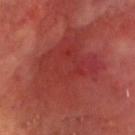workup: total-body-photography surveillance lesion; no biopsy
anatomic site: the head or neck
subject: male, in their mid-60s
acquisition: 15 mm crop, total-body photography